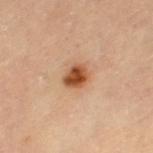The lesion was tiled from a total-body skin photograph and was not biopsied.
The lesion is located on the left thigh.
Longest diameter approximately 3 mm.
Cropped from a whole-body photographic skin survey; the tile spans about 15 mm.
The tile uses cross-polarized illumination.
A female subject in their 70s.